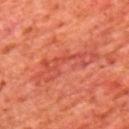Recorded during total-body skin imaging; not selected for excision or biopsy.
A region of skin cropped from a whole-body photographic capture, roughly 15 mm wide.
A male patient in their mid- to late 60s.
Located on the upper back.